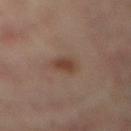No biopsy was performed on this lesion — it was imaged during a full skin examination and was not determined to be concerning.
Imaged with cross-polarized lighting.
From the mid back.
A male patient approximately 70 years of age.
A 15 mm close-up extracted from a 3D total-body photography capture.
The recorded lesion diameter is about 2.5 mm.